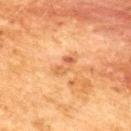Part of a total-body skin-imaging series; this lesion was reviewed on a skin check and was not flagged for biopsy. A male patient, roughly 50 years of age. The tile uses cross-polarized illumination. A 15 mm crop from a total-body photograph taken for skin-cancer surveillance. Located on the upper back.